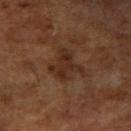subject: male, approximately 65 years of age
imaging modality: ~15 mm crop, total-body skin-cancer survey
lighting: cross-polarized
body site: the right upper arm
size: ~4 mm (longest diameter)